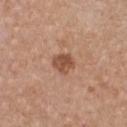| field | value |
|---|---|
| notes | total-body-photography surveillance lesion; no biopsy |
| subject | female, aged 33–37 |
| image-analysis metrics | an eccentricity of roughly 0.45 and a symmetry-axis asymmetry near 0.25; about 11 CIELAB-L* units darker than the surrounding skin; a nevus-likeness score of about 35/100 and lesion-presence confidence of about 100/100 |
| image source | ~15 mm tile from a whole-body skin photo |
| location | the chest |
| lesion diameter | ~3 mm (longest diameter) |